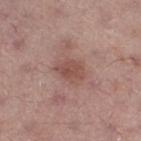The lesion is located on the right thigh. A male patient in their mid-50s. A close-up tile cropped from a whole-body skin photograph, about 15 mm across. Longest diameter approximately 3.5 mm. The lesion-visualizer software estimated radial color variation of about 1. It also reported a detector confidence of about 100 out of 100 that the crop contains a lesion.